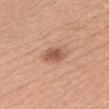Part of a total-body skin-imaging series; this lesion was reviewed on a skin check and was not flagged for biopsy. About 3.5 mm across. The lesion-visualizer software estimated a nevus-likeness score of about 80/100 and lesion-presence confidence of about 100/100. The subject is a female aged approximately 35. Cropped from a whole-body photographic skin survey; the tile spans about 15 mm. Captured under white-light illumination. The lesion is on the head or neck.